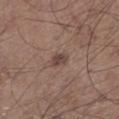Q: Was this lesion biopsied?
A: total-body-photography surveillance lesion; no biopsy
Q: How was this image acquired?
A: ~15 mm tile from a whole-body skin photo
Q: What lighting was used for the tile?
A: white-light
Q: Lesion size?
A: ≈2.5 mm
Q: Automated lesion metrics?
A: a footprint of about 3 mm², an outline eccentricity of about 0.8 (0 = round, 1 = elongated), and a shape-asymmetry score of about 0.25 (0 = symmetric); a lesion color around L≈43 a*≈16 b*≈21 in CIELAB, roughly 9 lightness units darker than nearby skin, and a normalized lesion–skin contrast near 7.5; a border-irregularity index near 2/10 and a peripheral color-asymmetry measure near 0.5
Q: What is the anatomic site?
A: the left lower leg
Q: Who is the patient?
A: male, about 75 years old